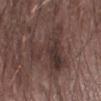This lesion was catalogued during total-body skin photography and was not selected for biopsy.
The subject is a male aged 73 to 77.
About 7.5 mm across.
This image is a 15 mm lesion crop taken from a total-body photograph.
On the arm.
Imaged with white-light lighting.